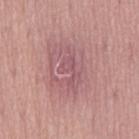Q: Who is the patient?
A: male, aged 53–57
Q: How was this image acquired?
A: ~15 mm crop, total-body skin-cancer survey
Q: Where on the body is the lesion?
A: the lower back
Q: What is the lesion's diameter?
A: about 3.5 mm